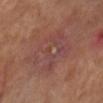A female patient, aged 63 to 67. The tile uses cross-polarized illumination. Longest diameter approximately 6 mm. The lesion is on the right forearm. A roughly 15 mm field-of-view crop from a total-body skin photograph. On biopsy, histopathology showed an actinic keratosis (borderline).